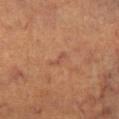Part of a total-body skin-imaging series; this lesion was reviewed on a skin check and was not flagged for biopsy.
The lesion is located on the right lower leg.
A female subject about 65 years old.
Cropped from a total-body skin-imaging series; the visible field is about 15 mm.
This is a cross-polarized tile.